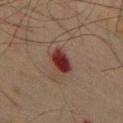<lesion>
  <biopsy_status>not biopsied; imaged during a skin examination</biopsy_status>
  <patient>
    <sex>male</sex>
    <age_approx>65</age_approx>
  </patient>
  <lesion_size>
    <long_diameter_mm_approx>4.0</long_diameter_mm_approx>
  </lesion_size>
  <lighting>cross-polarized</lighting>
  <image>
    <source>total-body photography crop</source>
    <field_of_view_mm>15</field_of_view_mm>
  </image>
  <site>back</site>
</lesion>